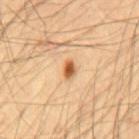Q: Is there a histopathology result?
A: catalogued during a skin exam; not biopsied
Q: Who is the patient?
A: male, in their mid-50s
Q: Automated lesion metrics?
A: border irregularity of about 2 on a 0–10 scale and a color-variation rating of about 3.5/10; a nevus-likeness score of about 100/100 and a detector confidence of about 100 out of 100 that the crop contains a lesion
Q: What is the anatomic site?
A: the mid back
Q: How was this image acquired?
A: ~15 mm tile from a whole-body skin photo
Q: Illumination type?
A: cross-polarized illumination